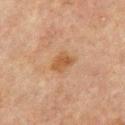Assessment: Part of a total-body skin-imaging series; this lesion was reviewed on a skin check and was not flagged for biopsy. Context: The patient is a male in their mid-60s. This is a cross-polarized tile. The lesion's longest dimension is about 3 mm. A 15 mm close-up tile from a total-body photography series done for melanoma screening. The lesion is on the upper back.